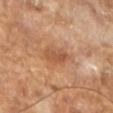Imaged during a routine full-body skin examination; the lesion was not biopsied and no histopathology is available.
Captured under cross-polarized illumination.
A 15 mm close-up extracted from a 3D total-body photography capture.
The patient is a male approximately 65 years of age.
Longest diameter approximately 3.5 mm.